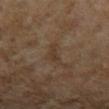Q: Is there a histopathology result?
A: total-body-photography surveillance lesion; no biopsy